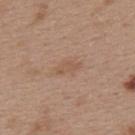Recorded during total-body skin imaging; not selected for excision or biopsy. Approximately 3 mm at its widest. A region of skin cropped from a whole-body photographic capture, roughly 15 mm wide. Located on the upper back. The patient is a female aged approximately 40. Captured under white-light illumination.